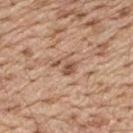Case summary:
• notes: no biopsy performed (imaged during a skin exam)
• anatomic site: the upper back
• illumination: white-light illumination
• image: ~15 mm crop, total-body skin-cancer survey
• subject: male, aged around 70